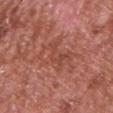workup: total-body-photography surveillance lesion; no biopsy
image: total-body-photography crop, ~15 mm field of view
tile lighting: white-light illumination
TBP lesion metrics: a lesion color around L≈48 a*≈27 b*≈29 in CIELAB, roughly 6 lightness units darker than nearby skin, and a normalized lesion–skin contrast near 5; a nevus-likeness score of about 0/100 and lesion-presence confidence of about 75/100
location: the front of the torso
lesion size: about 6.5 mm
patient: male, roughly 65 years of age